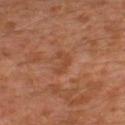Impression: This lesion was catalogued during total-body skin photography and was not selected for biopsy. Context: The lesion's longest dimension is about 3 mm. A lesion tile, about 15 mm wide, cut from a 3D total-body photograph. Captured under cross-polarized illumination. The lesion is located on the left thigh. The patient is a male about 30 years old.